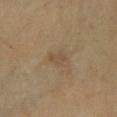Imaged during a routine full-body skin examination; the lesion was not biopsied and no histopathology is available.
Cropped from a whole-body photographic skin survey; the tile spans about 15 mm.
The tile uses cross-polarized illumination.
The subject is a male aged 58–62.
Located on the leg.
Longest diameter approximately 2.5 mm.